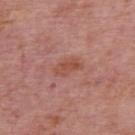Q: Was this lesion biopsied?
A: total-body-photography surveillance lesion; no biopsy
Q: What are the patient's age and sex?
A: male, aged 73 to 77
Q: What is the imaging modality?
A: 15 mm crop, total-body photography
Q: Lesion location?
A: the upper back